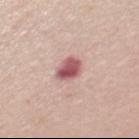Clinical impression:
The lesion was photographed on a routine skin check and not biopsied; there is no pathology result.
Background:
Imaged with white-light lighting. A 15 mm close-up tile from a total-body photography series done for melanoma screening. Automated tile analysis of the lesion measured a footprint of about 6.5 mm², an eccentricity of roughly 0.55, and a symmetry-axis asymmetry near 0.15. And it measured a mean CIELAB color near L≈56 a*≈28 b*≈19, a lesion–skin lightness drop of about 16, and a lesion-to-skin contrast of about 10.5 (normalized; higher = more distinct). The lesion is located on the chest. The patient is a female aged 33 to 37.